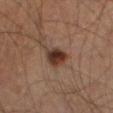No biopsy was performed on this lesion — it was imaged during a full skin examination and was not determined to be concerning.
Imaged with cross-polarized lighting.
The lesion is located on the left thigh.
A male subject aged 63 to 67.
A lesion tile, about 15 mm wide, cut from a 3D total-body photograph.
The recorded lesion diameter is about 3.5 mm.